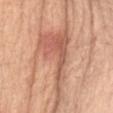Impression:
This lesion was catalogued during total-body skin photography and was not selected for biopsy.
Acquisition and patient details:
A female patient, in their mid-70s. The lesion's longest dimension is about 12.5 mm. Located on the abdomen. A close-up tile cropped from a whole-body skin photograph, about 15 mm across.